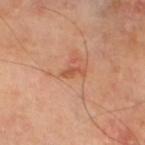Q: Was this lesion biopsied?
A: catalogued during a skin exam; not biopsied
Q: What is the anatomic site?
A: the left thigh
Q: Who is the patient?
A: male, about 65 years old
Q: What lighting was used for the tile?
A: cross-polarized illumination
Q: What is the imaging modality?
A: ~15 mm crop, total-body skin-cancer survey
Q: Automated lesion metrics?
A: an outline eccentricity of about 0.9 (0 = round, 1 = elongated) and two-axis asymmetry of about 0.45; a mean CIELAB color near L≈54 a*≈27 b*≈36, roughly 9 lightness units darker than nearby skin, and a normalized border contrast of about 6.5; a nevus-likeness score of about 0/100 and lesion-presence confidence of about 100/100